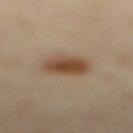Captured during whole-body skin photography for melanoma surveillance; the lesion was not biopsied. A 15 mm close-up extracted from a 3D total-body photography capture. On the leg. A female subject aged 33–37.